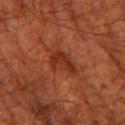Q: Was this lesion biopsied?
A: catalogued during a skin exam; not biopsied
Q: Lesion location?
A: the left lower leg
Q: How was this image acquired?
A: 15 mm crop, total-body photography
Q: Patient demographics?
A: male, aged 78 to 82
Q: What lighting was used for the tile?
A: cross-polarized illumination